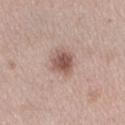No biopsy was performed on this lesion — it was imaged during a full skin examination and was not determined to be concerning.